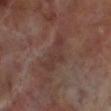Captured during whole-body skin photography for melanoma surveillance; the lesion was not biopsied.
A 15 mm close-up tile from a total-body photography series done for melanoma screening.
An algorithmic analysis of the crop reported a lesion area of about 8.5 mm², an eccentricity of roughly 0.9, and two-axis asymmetry of about 0.45. The software also gave a lesion color around L≈34 a*≈17 b*≈21 in CIELAB, roughly 6 lightness units darker than nearby skin, and a normalized border contrast of about 5.5. The analysis additionally found a within-lesion color-variation index near 2/10 and peripheral color asymmetry of about 0.5. It also reported a lesion-detection confidence of about 90/100.
The lesion is on the leg.
The lesion's longest dimension is about 5 mm.
The patient is a male aged approximately 70.